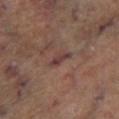Case summary:
– biopsy status: catalogued during a skin exam; not biopsied
– subject: male, in their mid- to late 60s
– site: the left lower leg
– image: ~15 mm tile from a whole-body skin photo